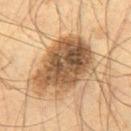Imaged during a routine full-body skin examination; the lesion was not biopsied and no histopathology is available. A close-up tile cropped from a whole-body skin photograph, about 15 mm across. From the leg. Imaged with cross-polarized lighting. A male subject aged around 60. The total-body-photography lesion software estimated a border-irregularity index near 3.5/10, internal color variation of about 7 on a 0–10 scale, and radial color variation of about 2.5. Longest diameter approximately 7.5 mm.